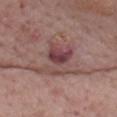Clinical impression: This lesion was catalogued during total-body skin photography and was not selected for biopsy. Context: Automated tile analysis of the lesion measured an area of roughly 24 mm², a shape eccentricity near 0.55, and a shape-asymmetry score of about 0.5 (0 = symmetric). And it measured a color-variation rating of about 8.5/10 and a peripheral color-asymmetry measure near 2. It also reported lesion-presence confidence of about 100/100. A female patient, aged 68–72. A 15 mm crop from a total-body photograph taken for skin-cancer surveillance. The lesion is on the back. Captured under white-light illumination.